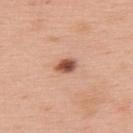<case>
  <biopsy_status>not biopsied; imaged during a skin examination</biopsy_status>
  <image>
    <source>total-body photography crop</source>
    <field_of_view_mm>15</field_of_view_mm>
  </image>
  <site>upper back</site>
  <patient>
    <sex>male</sex>
    <age_approx>60</age_approx>
  </patient>
  <lighting>white-light</lighting>
</case>